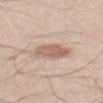biopsy status: catalogued during a skin exam; not biopsied
anatomic site: the mid back
subject: male, aged approximately 50
image-analysis metrics: a border-irregularity index near 2.5/10, a within-lesion color-variation index near 3/10, and a peripheral color-asymmetry measure near 1
acquisition: ~15 mm tile from a whole-body skin photo
size: about 4 mm